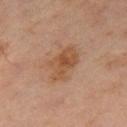  biopsy_status: not biopsied; imaged during a skin examination
  lighting: cross-polarized
  automated_metrics:
    area_mm2_approx: 9.5
    cielab_L: 43
    cielab_a: 18
    cielab_b: 30
    vs_skin_darker_L: 8.0
    vs_skin_contrast_norm: 7.5
    nevus_likeness_0_100: 25
    lesion_detection_confidence_0_100: 100
  lesion_size:
    long_diameter_mm_approx: 5.0
  image:
    source: total-body photography crop
    field_of_view_mm: 15
  site: leg
  patient:
    sex: female
    age_approx: 70